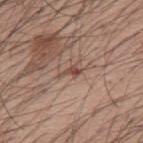Recorded during total-body skin imaging; not selected for excision or biopsy.
Imaged with white-light lighting.
About 3 mm across.
Automated image analysis of the tile measured a shape eccentricity near 0.85. The software also gave a border-irregularity index near 6.5/10, internal color variation of about 1.5 on a 0–10 scale, and peripheral color asymmetry of about 0.5. The software also gave a lesion-detection confidence of about 80/100.
A 15 mm close-up extracted from a 3D total-body photography capture.
The subject is a male in their 60s.
The lesion is on the back.